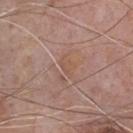Case summary:
– notes · imaged on a skin check; not biopsied
– body site · the front of the torso
– image · 15 mm crop, total-body photography
– subject · male, in their mid- to late 70s
– lighting · white-light